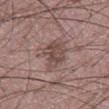Q: How was this image acquired?
A: ~15 mm tile from a whole-body skin photo
Q: What is the anatomic site?
A: the chest
Q: What is the lesion's diameter?
A: about 5 mm
Q: Illumination type?
A: white-light
Q: Patient demographics?
A: male, approximately 60 years of age